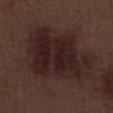This image is a 15 mm lesion crop taken from a total-body photograph. The lesion is on the leg. The recorded lesion diameter is about 9 mm. A male subject aged 68 to 72.